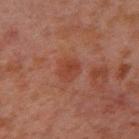Q: Is there a histopathology result?
A: total-body-photography surveillance lesion; no biopsy
Q: What is the anatomic site?
A: the arm
Q: What are the patient's age and sex?
A: male, aged approximately 30
Q: What lighting was used for the tile?
A: cross-polarized illumination
Q: What is the imaging modality?
A: ~15 mm tile from a whole-body skin photo